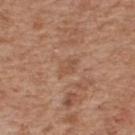Recorded during total-body skin imaging; not selected for excision or biopsy. A male subject about 65 years old. This is a white-light tile. From the back. Measured at roughly 2.5 mm in maximum diameter. Cropped from a total-body skin-imaging series; the visible field is about 15 mm.